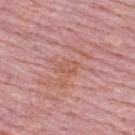Impression:
This lesion was catalogued during total-body skin photography and was not selected for biopsy.
Acquisition and patient details:
A male subject, aged 63–67. The lesion is on the upper back. A 15 mm close-up extracted from a 3D total-body photography capture.